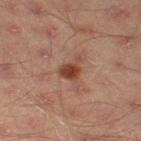Imaged during a routine full-body skin examination; the lesion was not biopsied and no histopathology is available.
Longest diameter approximately 3 mm.
The lesion is located on the left thigh.
A 15 mm crop from a total-body photograph taken for skin-cancer surveillance.
The subject is a male roughly 45 years of age.
The lesion-visualizer software estimated an average lesion color of about L≈34 a*≈20 b*≈25 (CIELAB), a lesion–skin lightness drop of about 10, and a normalized lesion–skin contrast near 9.5. It also reported a border-irregularity rating of about 3.5/10, a color-variation rating of about 2.5/10, and a peripheral color-asymmetry measure near 1.